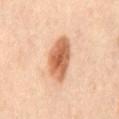Clinical impression: No biopsy was performed on this lesion — it was imaged during a full skin examination and was not determined to be concerning. Image and clinical context: On the mid back. This is a cross-polarized tile. This image is a 15 mm lesion crop taken from a total-body photograph. A male subject aged 63 to 67. Automated tile analysis of the lesion measured a lesion area of about 14 mm², an outline eccentricity of about 0.85 (0 = round, 1 = elongated), and a symmetry-axis asymmetry near 0.2. It also reported a mean CIELAB color near L≈63 a*≈25 b*≈37, a lesion–skin lightness drop of about 16, and a normalized border contrast of about 9.5. The software also gave a nevus-likeness score of about 100/100. The lesion's longest dimension is about 5.5 mm.